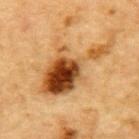* follow-up: total-body-photography surveillance lesion; no biopsy